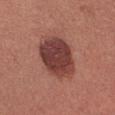Imaged during a routine full-body skin examination; the lesion was not biopsied and no histopathology is available. Located on the upper back. A female subject, in their mid- to late 30s. A region of skin cropped from a whole-body photographic capture, roughly 15 mm wide.